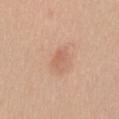biopsy status = imaged on a skin check; not biopsied | image source = ~15 mm crop, total-body skin-cancer survey | illumination = white-light illumination | image-analysis metrics = an average lesion color of about L≈61 a*≈22 b*≈31 (CIELAB), roughly 8 lightness units darker than nearby skin, and a normalized lesion–skin contrast near 5; border irregularity of about 4 on a 0–10 scale, internal color variation of about 1 on a 0–10 scale, and peripheral color asymmetry of about 0.5; a nevus-likeness score of about 30/100 | subject = male, aged approximately 40 | diameter = about 3 mm | location = the chest.